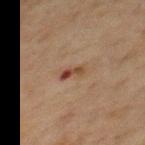follow-up = catalogued during a skin exam; not biopsied.